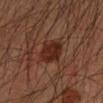The lesion was tiled from a total-body skin photograph and was not biopsied.
The patient is a male in their mid-30s.
Automated tile analysis of the lesion measured a lesion area of about 8.5 mm² and a symmetry-axis asymmetry near 0.2. It also reported a border-irregularity index near 2/10, a within-lesion color-variation index near 3.5/10, and radial color variation of about 1.
Imaged with cross-polarized lighting.
The lesion is on the left forearm.
A lesion tile, about 15 mm wide, cut from a 3D total-body photograph.